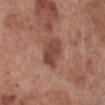Impression: The lesion was photographed on a routine skin check and not biopsied; there is no pathology result. Image and clinical context: The lesion is located on the leg. Cropped from a total-body skin-imaging series; the visible field is about 15 mm. A male patient, approximately 70 years of age.